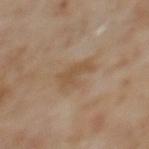Clinical impression: The lesion was tiled from a total-body skin photograph and was not biopsied. Acquisition and patient details: A female patient roughly 60 years of age. Cropped from a whole-body photographic skin survey; the tile spans about 15 mm. The lesion's longest dimension is about 4 mm. The total-body-photography lesion software estimated a shape-asymmetry score of about 0.4 (0 = symmetric). It also reported an average lesion color of about L≈50 a*≈15 b*≈31 (CIELAB) and a lesion–skin lightness drop of about 7. The analysis additionally found a border-irregularity index near 5/10 and radial color variation of about 0.5. Imaged with cross-polarized lighting. From the upper back.